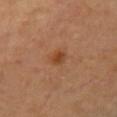Case summary:
– workup: imaged on a skin check; not biopsied
– patient: female, aged around 40
– tile lighting: cross-polarized
– automated metrics: a footprint of about 2.5 mm², an outline eccentricity of about 0.6 (0 = round, 1 = elongated), and a shape-asymmetry score of about 0.2 (0 = symmetric); a lesion color around L≈43 a*≈23 b*≈36 in CIELAB; a border-irregularity rating of about 1.5/10, a color-variation rating of about 3/10, and peripheral color asymmetry of about 1
– body site: the chest
– acquisition: ~15 mm crop, total-body skin-cancer survey